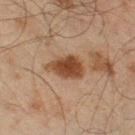Assessment: Imaged during a routine full-body skin examination; the lesion was not biopsied and no histopathology is available. Context: A 15 mm crop from a total-body photograph taken for skin-cancer surveillance. A male patient, aged around 45. Imaged with cross-polarized lighting. The lesion is on the right thigh.